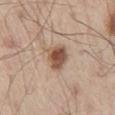follow-up: imaged on a skin check; not biopsied | location: the right thigh | subject: male, approximately 60 years of age | acquisition: ~15 mm tile from a whole-body skin photo.